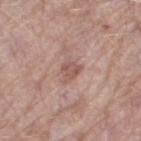Part of a total-body skin-imaging series; this lesion was reviewed on a skin check and was not flagged for biopsy. Automated image analysis of the tile measured a lesion area of about 4 mm² and an eccentricity of roughly 0.65. It also reported an average lesion color of about L≈54 a*≈22 b*≈23 (CIELAB), a lesion–skin lightness drop of about 9, and a normalized border contrast of about 6.5. And it measured a border-irregularity rating of about 2.5/10, a within-lesion color-variation index near 2/10, and peripheral color asymmetry of about 0.5. A lesion tile, about 15 mm wide, cut from a 3D total-body photograph. The recorded lesion diameter is about 3 mm. The patient is a female aged 68 to 72. The lesion is on the right thigh.